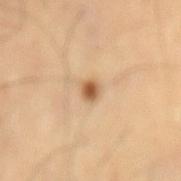| key | value |
|---|---|
| notes | no biopsy performed (imaged during a skin exam) |
| acquisition | 15 mm crop, total-body photography |
| TBP lesion metrics | a lesion color around L≈56 a*≈19 b*≈36 in CIELAB, roughly 14 lightness units darker than nearby skin, and a lesion-to-skin contrast of about 9 (normalized; higher = more distinct); a nevus-likeness score of about 95/100 and lesion-presence confidence of about 100/100 |
| location | the back |
| tile lighting | cross-polarized |
| diameter | ≈2 mm |
| subject | male, about 60 years old |